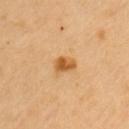biopsy status: no biopsy performed (imaged during a skin exam) | illumination: cross-polarized | size: ≈2.5 mm | acquisition: 15 mm crop, total-body photography | subject: female, approximately 60 years of age | site: the left upper arm.